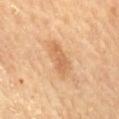subject = male, aged 83 or over; image source = ~15 mm tile from a whole-body skin photo; site = the chest.